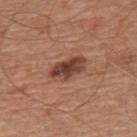follow-up — catalogued during a skin exam; not biopsied
site — the mid back
illumination — white-light
subject — male, approximately 65 years of age
image source — total-body-photography crop, ~15 mm field of view